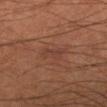biopsy_status: not biopsied; imaged during a skin examination
image:
  source: total-body photography crop
  field_of_view_mm: 15
site: left lower leg
lesion_size:
  long_diameter_mm_approx: 3.0
patient:
  sex: male
  age_approx: 55
lighting: cross-polarized
automated_metrics:
  area_mm2_approx: 3.5
  eccentricity: 0.9
  shape_asymmetry: 0.4
  cielab_L: 36
  cielab_a: 20
  cielab_b: 25
  vs_skin_darker_L: 5.0
  vs_skin_contrast_norm: 4.5
  border_irregularity_0_10: 5.0
  color_variation_0_10: 0.5
  peripheral_color_asymmetry: 0.0
  nevus_likeness_0_100: 0
  lesion_detection_confidence_0_100: 95